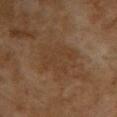Clinical impression: Part of a total-body skin-imaging series; this lesion was reviewed on a skin check and was not flagged for biopsy. Background: The lesion is located on the upper back. Automated image analysis of the tile measured a lesion area of about 15 mm², an eccentricity of roughly 0.7, and a shape-asymmetry score of about 0.3 (0 = symmetric). The analysis additionally found radial color variation of about 1. The software also gave a nevus-likeness score of about 0/100 and lesion-presence confidence of about 100/100. A female patient, aged approximately 60. A lesion tile, about 15 mm wide, cut from a 3D total-body photograph. Imaged with cross-polarized lighting.